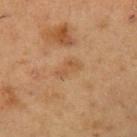Assessment:
The lesion was photographed on a routine skin check and not biopsied; there is no pathology result.
Image and clinical context:
A male subject, about 55 years old. About 3.5 mm across. Cropped from a total-body skin-imaging series; the visible field is about 15 mm. The lesion is located on the left upper arm. The total-body-photography lesion software estimated a lesion area of about 4 mm², a shape eccentricity near 0.9, and two-axis asymmetry of about 0.4. And it measured lesion-presence confidence of about 100/100.